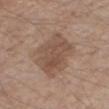notes: catalogued during a skin exam; not biopsied | patient: male, aged 63–67 | illumination: white-light illumination | automated lesion analysis: a border-irregularity rating of about 2.5/10, a within-lesion color-variation index near 3/10, and a peripheral color-asymmetry measure near 1 | acquisition: ~15 mm crop, total-body skin-cancer survey | diameter: ~6 mm (longest diameter) | body site: the right thigh.